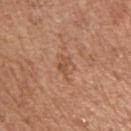| key | value |
|---|---|
| follow-up | total-body-photography surveillance lesion; no biopsy |
| site | the left upper arm |
| diameter | about 3 mm |
| imaging modality | total-body-photography crop, ~15 mm field of view |
| patient | female, in their mid-70s |